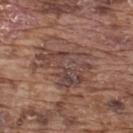The patient is a male aged approximately 75. This image is a 15 mm lesion crop taken from a total-body photograph. The lesion is on the upper back.